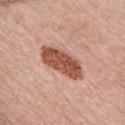Part of a total-body skin-imaging series; this lesion was reviewed on a skin check and was not flagged for biopsy.
An algorithmic analysis of the crop reported a border-irregularity rating of about 2.5/10, a color-variation rating of about 4/10, and radial color variation of about 1.5. It also reported a classifier nevus-likeness of about 95/100 and a detector confidence of about 100 out of 100 that the crop contains a lesion.
A lesion tile, about 15 mm wide, cut from a 3D total-body photograph.
Located on the chest.
A male subject roughly 60 years of age.
Measured at roughly 6 mm in maximum diameter.
Imaged with white-light lighting.